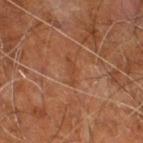workup=imaged on a skin check; not biopsied
illumination=cross-polarized illumination
imaging modality=~15 mm crop, total-body skin-cancer survey
lesion size=about 3 mm
patient=male, aged 58 to 62
anatomic site=the right leg